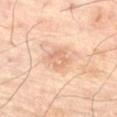Assessment: The lesion was tiled from a total-body skin photograph and was not biopsied. Clinical summary: Automated image analysis of the tile measured a lesion area of about 4.5 mm² and an outline eccentricity of about 0.65 (0 = round, 1 = elongated). The recorded lesion diameter is about 2.5 mm. The tile uses cross-polarized illumination. The lesion is located on the right thigh. A male subject aged around 70. Cropped from a whole-body photographic skin survey; the tile spans about 15 mm.